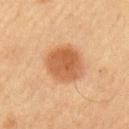Assessment:
Recorded during total-body skin imaging; not selected for excision or biopsy.
Clinical summary:
A male subject aged approximately 65. Captured under cross-polarized illumination. Automated tile analysis of the lesion measured an area of roughly 15 mm². A lesion tile, about 15 mm wide, cut from a 3D total-body photograph. Measured at roughly 4.5 mm in maximum diameter. On the right upper arm.